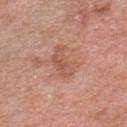workup = no biopsy performed (imaged during a skin exam) | imaging modality = ~15 mm tile from a whole-body skin photo | location = the chest | subject = male, about 70 years old | image-analysis metrics = an average lesion color of about L≈55 a*≈23 b*≈31 (CIELAB); a nevus-likeness score of about 0/100 and a detector confidence of about 100 out of 100 that the crop contains a lesion.